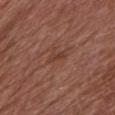No biopsy was performed on this lesion — it was imaged during a full skin examination and was not determined to be concerning.
From the chest.
A female subject aged 73–77.
Captured under white-light illumination.
About 2.5 mm across.
A close-up tile cropped from a whole-body skin photograph, about 15 mm across.